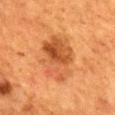Case summary:
* notes: catalogued during a skin exam; not biopsied
* image source: ~15 mm tile from a whole-body skin photo
* lesion diameter: ~6 mm (longest diameter)
* patient: female, in their mid-50s
* location: the mid back